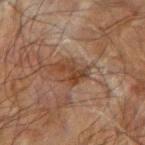Case summary:
- notes — catalogued during a skin exam; not biopsied
- image source — 15 mm crop, total-body photography
- diameter — about 4 mm
- lighting — cross-polarized illumination
- patient — male, aged around 70
- location — the left forearm
- image-analysis metrics — an area of roughly 4.5 mm², an eccentricity of roughly 0.9, and a symmetry-axis asymmetry near 0.6; a classifier nevus-likeness of about 0/100 and lesion-presence confidence of about 100/100